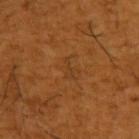Assessment: The lesion was photographed on a routine skin check and not biopsied; there is no pathology result. Clinical summary: A male patient approximately 65 years of age. Automated tile analysis of the lesion measured a classifier nevus-likeness of about 0/100. Located on the left upper arm. Captured under cross-polarized illumination. This image is a 15 mm lesion crop taken from a total-body photograph. The lesion's longest dimension is about 2.5 mm.